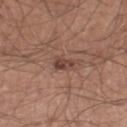Captured during whole-body skin photography for melanoma surveillance; the lesion was not biopsied.
Longest diameter approximately 3 mm.
The lesion is on the left thigh.
This is a white-light tile.
The patient is a male aged around 40.
A region of skin cropped from a whole-body photographic capture, roughly 15 mm wide.
The total-body-photography lesion software estimated a lesion area of about 4 mm², an outline eccentricity of about 0.85 (0 = round, 1 = elongated), and two-axis asymmetry of about 0.3. The analysis additionally found a mean CIELAB color near L≈43 a*≈20 b*≈24, a lesion–skin lightness drop of about 10, and a lesion-to-skin contrast of about 7.5 (normalized; higher = more distinct). It also reported a border-irregularity rating of about 3/10, internal color variation of about 4 on a 0–10 scale, and a peripheral color-asymmetry measure near 1.5.